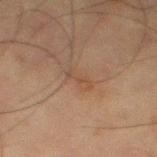The lesion was photographed on a routine skin check and not biopsied; there is no pathology result. Imaged with cross-polarized lighting. A region of skin cropped from a whole-body photographic capture, roughly 15 mm wide. On the leg. A male patient, about 65 years old.